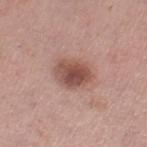biopsy status = imaged on a skin check; not biopsied
body site = the right thigh
lesion diameter = ≈4.5 mm
subject = female, in their 40s
imaging modality = 15 mm crop, total-body photography